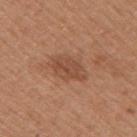Imaged with white-light lighting. The patient is a male aged around 30. The lesion is on the right upper arm. Automated image analysis of the tile measured border irregularity of about 2.5 on a 0–10 scale and internal color variation of about 3.5 on a 0–10 scale. And it measured lesion-presence confidence of about 100/100. The lesion's longest dimension is about 4 mm. This image is a 15 mm lesion crop taken from a total-body photograph.